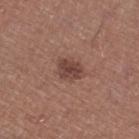Part of a total-body skin-imaging series; this lesion was reviewed on a skin check and was not flagged for biopsy. A 15 mm crop from a total-body photograph taken for skin-cancer surveillance. This is a white-light tile. About 3 mm across. The lesion is on the right thigh. The patient is a male approximately 65 years of age.